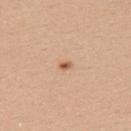  biopsy_status: not biopsied; imaged during a skin examination
  site: left upper arm
  image:
    source: total-body photography crop
    field_of_view_mm: 15
  automated_metrics:
    area_mm2_approx: 1.5
    eccentricity: 0.75
    shape_asymmetry: 0.3
    border_irregularity_0_10: 2.0
    peripheral_color_asymmetry: 0.0
    nevus_likeness_0_100: 90
    lesion_detection_confidence_0_100: 100
  lighting: white-light
  patient:
    sex: female
    age_approx: 25